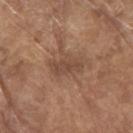The lesion was tiled from a total-body skin photograph and was not biopsied.
The lesion is located on the right upper arm.
The lesion's longest dimension is about 3.5 mm.
A roughly 15 mm field-of-view crop from a total-body skin photograph.
The patient is a male in their 80s.